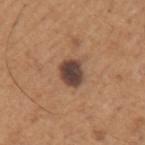| key | value |
|---|---|
| notes | catalogued during a skin exam; not biopsied |
| location | the left upper arm |
| image | ~15 mm tile from a whole-body skin photo |
| patient | male, aged around 65 |
| lesion diameter | ≈3.5 mm |